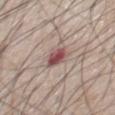{
  "biopsy_status": "not biopsied; imaged during a skin examination"
}